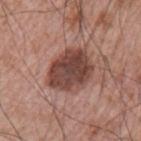Q: Was a biopsy performed?
A: catalogued during a skin exam; not biopsied
Q: Patient demographics?
A: male, aged approximately 65
Q: What did automated image analysis measure?
A: a footprint of about 20 mm², an outline eccentricity of about 0.6 (0 = round, 1 = elongated), and a shape-asymmetry score of about 0.15 (0 = symmetric); a border-irregularity rating of about 1.5/10, internal color variation of about 5 on a 0–10 scale, and a peripheral color-asymmetry measure near 1.5; a lesion-detection confidence of about 100/100
Q: What is the lesion's diameter?
A: ~5.5 mm (longest diameter)
Q: How was this image acquired?
A: ~15 mm tile from a whole-body skin photo
Q: Illumination type?
A: white-light illumination
Q: Where on the body is the lesion?
A: the left upper arm